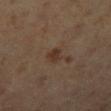The lesion was tiled from a total-body skin photograph and was not biopsied. A female patient, in their mid- to late 50s. An algorithmic analysis of the crop reported a border-irregularity rating of about 2.5/10, internal color variation of about 3.5 on a 0–10 scale, and peripheral color asymmetry of about 1.5. This image is a 15 mm lesion crop taken from a total-body photograph. Longest diameter approximately 2.5 mm. Located on the right lower leg. Imaged with cross-polarized lighting.